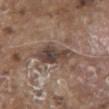Imaged during a routine full-body skin examination; the lesion was not biopsied and no histopathology is available.
A roughly 15 mm field-of-view crop from a total-body skin photograph.
From the mid back.
A male patient, aged 78–82.